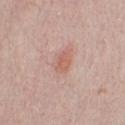This lesion was catalogued during total-body skin photography and was not selected for biopsy. A male patient aged around 25. A close-up tile cropped from a whole-body skin photograph, about 15 mm across. The lesion is on the chest. About 3 mm across. Imaged with white-light lighting.